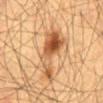Part of a total-body skin-imaging series; this lesion was reviewed on a skin check and was not flagged for biopsy. Cropped from a total-body skin-imaging series; the visible field is about 15 mm. Longest diameter approximately 7 mm. The total-body-photography lesion software estimated an outline eccentricity of about 0.9 (0 = round, 1 = elongated) and two-axis asymmetry of about 0.5. It also reported a mean CIELAB color near L≈53 a*≈21 b*≈37, a lesion–skin lightness drop of about 13, and a lesion-to-skin contrast of about 8.5 (normalized; higher = more distinct). The lesion is on the abdomen. The subject is a male approximately 60 years of age.